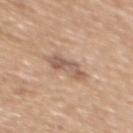Acquisition and patient details: On the upper back. A close-up tile cropped from a whole-body skin photograph, about 15 mm across. The subject is a female approximately 55 years of age. This is a white-light tile.